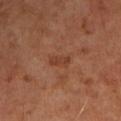Context: A male subject, roughly 65 years of age. Captured under cross-polarized illumination. The lesion is on the left upper arm. Longest diameter approximately 3 mm. A region of skin cropped from a whole-body photographic capture, roughly 15 mm wide. An algorithmic analysis of the crop reported an area of roughly 4 mm², an eccentricity of roughly 0.85, and a symmetry-axis asymmetry near 0.3.